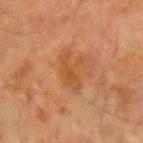Captured during whole-body skin photography for melanoma surveillance; the lesion was not biopsied.
The lesion is on the arm.
A male subject, aged approximately 65.
The recorded lesion diameter is about 4 mm.
A roughly 15 mm field-of-view crop from a total-body skin photograph.